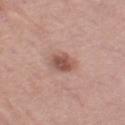{
  "biopsy_status": "not biopsied; imaged during a skin examination",
  "automated_metrics": {
    "area_mm2_approx": 6.0,
    "shape_asymmetry": 0.15,
    "cielab_L": 53,
    "cielab_a": 22,
    "cielab_b": 26,
    "vs_skin_darker_L": 12.0,
    "border_irregularity_0_10": 1.5,
    "color_variation_0_10": 4.0,
    "nevus_likeness_0_100": 85,
    "lesion_detection_confidence_0_100": 100
  },
  "site": "left thigh",
  "lighting": "white-light",
  "patient": {
    "sex": "female",
    "age_approx": 50
  },
  "image": {
    "source": "total-body photography crop",
    "field_of_view_mm": 15
  }
}